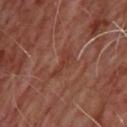Impression:
Part of a total-body skin-imaging series; this lesion was reviewed on a skin check and was not flagged for biopsy.
Clinical summary:
Automated tile analysis of the lesion measured a footprint of about 4.5 mm², an outline eccentricity of about 0.9 (0 = round, 1 = elongated), and two-axis asymmetry of about 0.5. It also reported a border-irregularity rating of about 5.5/10, internal color variation of about 1.5 on a 0–10 scale, and a peripheral color-asymmetry measure near 0.5. The lesion's longest dimension is about 4 mm. A male subject aged 63 to 67. A 15 mm close-up tile from a total-body photography series done for melanoma screening. On the back. The tile uses cross-polarized illumination.